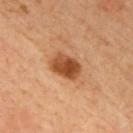Assessment:
Imaged during a routine full-body skin examination; the lesion was not biopsied and no histopathology is available.
Acquisition and patient details:
The lesion is located on the chest. Approximately 3.5 mm at its widest. A 15 mm close-up extracted from a 3D total-body photography capture. Automated tile analysis of the lesion measured a lesion–skin lightness drop of about 14 and a normalized border contrast of about 10.5. The analysis additionally found lesion-presence confidence of about 100/100. The patient is a male aged around 65.